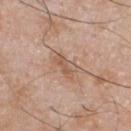| field | value |
|---|---|
| biopsy status | no biopsy performed (imaged during a skin exam) |
| acquisition | ~15 mm crop, total-body skin-cancer survey |
| anatomic site | the chest |
| patient | male, aged approximately 50 |
| automated metrics | border irregularity of about 3.5 on a 0–10 scale and internal color variation of about 3 on a 0–10 scale; lesion-presence confidence of about 100/100 |
| size | ~3.5 mm (longest diameter) |